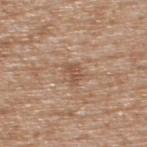Q: Is there a histopathology result?
A: total-body-photography surveillance lesion; no biopsy